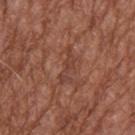This lesion was catalogued during total-body skin photography and was not selected for biopsy. The subject is a male in their mid-70s. On the upper back. Captured under white-light illumination. About 4.5 mm across. A roughly 15 mm field-of-view crop from a total-body skin photograph.